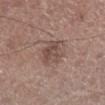Recorded during total-body skin imaging; not selected for excision or biopsy.
The lesion's longest dimension is about 4 mm.
Located on the right lower leg.
A male patient, in their mid- to late 50s.
Imaged with white-light lighting.
Automated image analysis of the tile measured a lesion color around L≈48 a*≈17 b*≈23 in CIELAB, about 9 CIELAB-L* units darker than the surrounding skin, and a lesion-to-skin contrast of about 6.5 (normalized; higher = more distinct).
A lesion tile, about 15 mm wide, cut from a 3D total-body photograph.